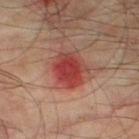| field | value |
|---|---|
| notes | total-body-photography surveillance lesion; no biopsy |
| subject | male, aged 73–77 |
| tile lighting | cross-polarized illumination |
| image source | ~15 mm crop, total-body skin-cancer survey |
| automated metrics | a footprint of about 10 mm², a shape eccentricity near 0.5, and a symmetry-axis asymmetry near 0.2; a lesion color around L≈35 a*≈29 b*≈24 in CIELAB and a normalized lesion–skin contrast near 9; a border-irregularity rating of about 2/10, internal color variation of about 4 on a 0–10 scale, and peripheral color asymmetry of about 1.5; an automated nevus-likeness rating near 0 out of 100 and a detector confidence of about 100 out of 100 that the crop contains a lesion |
| site | the right thigh |
| lesion diameter | ~4 mm (longest diameter) |